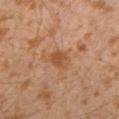| field | value |
|---|---|
| biopsy status | catalogued during a skin exam; not biopsied |
| patient | male, aged around 30 |
| body site | the left lower leg |
| image | 15 mm crop, total-body photography |
| illumination | cross-polarized illumination |
| automated metrics | a footprint of about 5 mm², an outline eccentricity of about 0.8 (0 = round, 1 = elongated), and two-axis asymmetry of about 0.15; a border-irregularity rating of about 2/10, a within-lesion color-variation index near 4/10, and peripheral color asymmetry of about 1.5 |
| size | ≈3 mm |